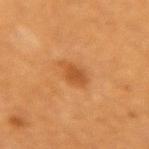workup=total-body-photography surveillance lesion; no biopsy